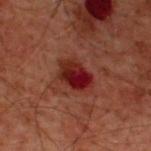| feature | finding |
|---|---|
| notes | no biopsy performed (imaged during a skin exam) |